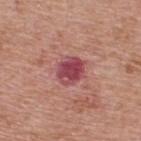- workup: total-body-photography surveillance lesion; no biopsy
- site: the upper back
- subject: male, approximately 70 years of age
- image: ~15 mm tile from a whole-body skin photo
- lighting: white-light illumination
- diameter: ~3.5 mm (longest diameter)